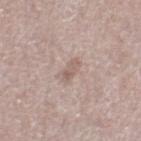Recorded during total-body skin imaging; not selected for excision or biopsy. Longest diameter approximately 2.5 mm. From the leg. Cropped from a total-body skin-imaging series; the visible field is about 15 mm. A female patient aged around 65.